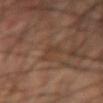Clinical impression: Captured during whole-body skin photography for melanoma surveillance; the lesion was not biopsied. Clinical summary: A male subject about 65 years old. Captured under cross-polarized illumination. The lesion is on the left forearm. A roughly 15 mm field-of-view crop from a total-body skin photograph.